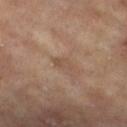{"biopsy_status": "not biopsied; imaged during a skin examination", "site": "right lower leg", "patient": {"sex": "female", "age_approx": 75}, "image": {"source": "total-body photography crop", "field_of_view_mm": 15}}